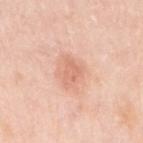Part of a total-body skin-imaging series; this lesion was reviewed on a skin check and was not flagged for biopsy.
Imaged with white-light lighting.
A female patient aged 63–67.
A roughly 15 mm field-of-view crop from a total-body skin photograph.
The lesion is located on the right upper arm.